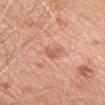Findings:
– follow-up — total-body-photography surveillance lesion; no biopsy
– acquisition — ~15 mm crop, total-body skin-cancer survey
– anatomic site — the chest
– diameter — ≈2.5 mm
– automated lesion analysis — an area of roughly 3.5 mm², a shape eccentricity near 0.7, and two-axis asymmetry of about 0.35; a lesion color around L≈61 a*≈25 b*≈34 in CIELAB and a lesion-to-skin contrast of about 6 (normalized; higher = more distinct)
– tile lighting — white-light illumination
– subject — female, roughly 65 years of age